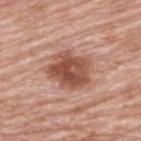Notes:
– anatomic site · the upper back
– image · total-body-photography crop, ~15 mm field of view
– subject · male, approximately 80 years of age